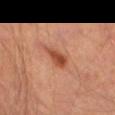Part of a total-body skin-imaging series; this lesion was reviewed on a skin check and was not flagged for biopsy.
From the leg.
A lesion tile, about 15 mm wide, cut from a 3D total-body photograph.
A male patient, roughly 65 years of age.
Imaged with cross-polarized lighting.
About 3.5 mm across.